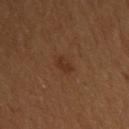Q: Was a biopsy performed?
A: no biopsy performed (imaged during a skin exam)
Q: Automated lesion metrics?
A: a lesion color around L≈26 a*≈17 b*≈25 in CIELAB, roughly 5 lightness units darker than nearby skin, and a normalized lesion–skin contrast near 5.5; a within-lesion color-variation index near 1.5/10 and radial color variation of about 0.5
Q: What kind of image is this?
A: total-body-photography crop, ~15 mm field of view
Q: What is the anatomic site?
A: the upper back
Q: How large is the lesion?
A: ≈2.5 mm
Q: Illumination type?
A: cross-polarized
Q: Patient demographics?
A: female, aged 48 to 52